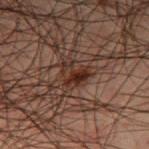Clinical impression: No biopsy was performed on this lesion — it was imaged during a full skin examination and was not determined to be concerning. Acquisition and patient details: The lesion's longest dimension is about 3 mm. A 15 mm close-up extracted from a 3D total-body photography capture. A male patient aged approximately 50. Located on the right thigh. Captured under cross-polarized illumination.